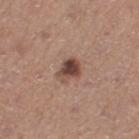This lesion was catalogued during total-body skin photography and was not selected for biopsy. A 15 mm crop from a total-body photograph taken for skin-cancer surveillance. A female subject approximately 55 years of age. The tile uses white-light illumination. From the leg.